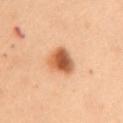biopsy status = catalogued during a skin exam; not biopsied | illumination = cross-polarized | patient = female, in their 50s | location = the mid back | image source = ~15 mm tile from a whole-body skin photo | size = about 4 mm | image-analysis metrics = an area of roughly 8.5 mm², an eccentricity of roughly 0.7, and two-axis asymmetry of about 0.3; a classifier nevus-likeness of about 100/100 and a detector confidence of about 100 out of 100 that the crop contains a lesion.